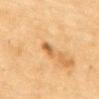The lesion was photographed on a routine skin check and not biopsied; there is no pathology result.
Located on the upper back.
A female patient, in their 80s.
This is a cross-polarized tile.
Cropped from a whole-body photographic skin survey; the tile spans about 15 mm.
Automated image analysis of the tile measured a lesion area of about 3.5 mm², a shape eccentricity near 0.9, and a shape-asymmetry score of about 0.25 (0 = symmetric). It also reported an average lesion color of about L≈53 a*≈20 b*≈39 (CIELAB), a lesion–skin lightness drop of about 10, and a normalized border contrast of about 7.5. It also reported a nevus-likeness score of about 40/100.